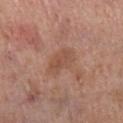biopsy_status: not biopsied; imaged during a skin examination
patient:
  sex: male
  age_approx: 70
image:
  source: total-body photography crop
  field_of_view_mm: 15
lesion_size:
  long_diameter_mm_approx: 4.0
lighting: white-light
automated_metrics:
  cielab_L: 51
  cielab_a: 21
  cielab_b: 29
  vs_skin_darker_L: 7.0
  border_irregularity_0_10: 2.5
  color_variation_0_10: 2.5
  peripheral_color_asymmetry: 1.0
site: right lower leg